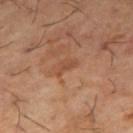Clinical impression:
The lesion was tiled from a total-body skin photograph and was not biopsied.
Acquisition and patient details:
From the right thigh. Approximately 3 mm at its widest. The lesion-visualizer software estimated a lesion area of about 2.5 mm², an outline eccentricity of about 0.9 (0 = round, 1 = elongated), and two-axis asymmetry of about 0.5. And it measured an average lesion color of about L≈48 a*≈23 b*≈32 (CIELAB), a lesion–skin lightness drop of about 7, and a lesion-to-skin contrast of about 5.5 (normalized; higher = more distinct). The analysis additionally found a border-irregularity index near 4.5/10, internal color variation of about 0.5 on a 0–10 scale, and peripheral color asymmetry of about 0. It also reported a classifier nevus-likeness of about 0/100 and a detector confidence of about 100 out of 100 that the crop contains a lesion. A male patient aged 63 to 67. Imaged with cross-polarized lighting. Cropped from a total-body skin-imaging series; the visible field is about 15 mm.